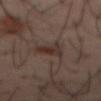Impression:
The lesion was tiled from a total-body skin photograph and was not biopsied.
Image and clinical context:
A 15 mm crop from a total-body photograph taken for skin-cancer surveillance. The lesion's longest dimension is about 3.5 mm. A male subject roughly 40 years of age. The lesion is on the abdomen.